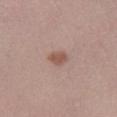Notes:
* biopsy status: no biopsy performed (imaged during a skin exam)
* site: the right lower leg
* subject: male, approximately 35 years of age
* illumination: white-light illumination
* acquisition: 15 mm crop, total-body photography
* size: ≈2.5 mm
* automated lesion analysis: a symmetry-axis asymmetry near 0.15; a mean CIELAB color near L≈53 a*≈20 b*≈25 and a normalized lesion–skin contrast near 7.5; a border-irregularity index near 1.5/10, a color-variation rating of about 1/10, and a peripheral color-asymmetry measure near 0.5